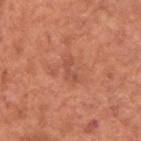The lesion was tiled from a total-body skin photograph and was not biopsied.
From the back.
An algorithmic analysis of the crop reported an area of roughly 2 mm², an outline eccentricity of about 0.9 (0 = round, 1 = elongated), and two-axis asymmetry of about 0.55. And it measured a border-irregularity index near 7/10. The analysis additionally found an automated nevus-likeness rating near 0 out of 100 and a detector confidence of about 100 out of 100 that the crop contains a lesion.
A male subject, roughly 65 years of age.
Longest diameter approximately 2.5 mm.
A 15 mm crop from a total-body photograph taken for skin-cancer surveillance.
Captured under white-light illumination.